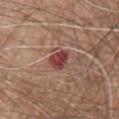The lesion was photographed on a routine skin check and not biopsied; there is no pathology result. Captured under white-light illumination. Measured at roughly 3 mm in maximum diameter. Automated image analysis of the tile measured a shape eccentricity near 0.55 and a shape-asymmetry score of about 0.2 (0 = symmetric). It also reported a normalized lesion–skin contrast near 9.5. And it measured a border-irregularity rating of about 2/10, a within-lesion color-variation index near 4/10, and peripheral color asymmetry of about 1.5. It also reported a classifier nevus-likeness of about 0/100. The subject is a male aged 78 to 82. A 15 mm crop from a total-body photograph taken for skin-cancer surveillance. The lesion is on the front of the torso.